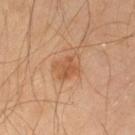workup: total-body-photography surveillance lesion; no biopsy
TBP lesion metrics: a lesion area of about 7 mm² and an eccentricity of roughly 0.7; a lesion color around L≈51 a*≈21 b*≈34 in CIELAB, a lesion–skin lightness drop of about 8, and a lesion-to-skin contrast of about 6 (normalized; higher = more distinct)
acquisition: 15 mm crop, total-body photography
diameter: ~3.5 mm (longest diameter)
subject: male, aged approximately 60
anatomic site: the left thigh
illumination: cross-polarized